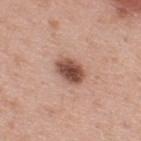Q: Is there a histopathology result?
A: imaged on a skin check; not biopsied
Q: Lesion size?
A: ≈3.5 mm
Q: What is the imaging modality?
A: 15 mm crop, total-body photography
Q: Patient demographics?
A: male, aged approximately 40
Q: What is the anatomic site?
A: the upper back
Q: What lighting was used for the tile?
A: white-light
Q: Automated lesion metrics?
A: a lesion color around L≈50 a*≈22 b*≈27 in CIELAB, roughly 16 lightness units darker than nearby skin, and a lesion-to-skin contrast of about 11 (normalized; higher = more distinct)